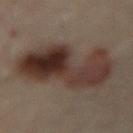workup — total-body-photography surveillance lesion; no biopsy
image — ~15 mm tile from a whole-body skin photo
body site — the lower back
lighting — cross-polarized illumination
diameter — ≈10 mm
subject — female, aged 58 to 62